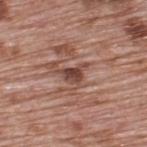Captured during whole-body skin photography for melanoma surveillance; the lesion was not biopsied.
Captured under white-light illumination.
On the upper back.
A male patient aged around 70.
A close-up tile cropped from a whole-body skin photograph, about 15 mm across.
Approximately 3.5 mm at its widest.